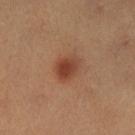follow-up=no biopsy performed (imaged during a skin exam) | lesion diameter=about 3.5 mm | patient=female, roughly 40 years of age | image-analysis metrics=a shape eccentricity near 0.65; a border-irregularity rating of about 1.5/10, a color-variation rating of about 3.5/10, and peripheral color asymmetry of about 1; lesion-presence confidence of about 100/100 | imaging modality=~15 mm tile from a whole-body skin photo | location=the left lower leg.